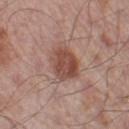biopsy status: no biopsy performed (imaged during a skin exam).